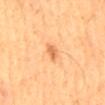{
  "biopsy_status": "not biopsied; imaged during a skin examination",
  "patient": {
    "sex": "male",
    "age_approx": 60
  },
  "lighting": "cross-polarized",
  "site": "back",
  "automated_metrics": {
    "area_mm2_approx": 2.5,
    "cielab_L": 66,
    "cielab_a": 27,
    "cielab_b": 44,
    "vs_skin_contrast_norm": 7.0,
    "border_irregularity_0_10": 2.0,
    "color_variation_0_10": 1.5,
    "peripheral_color_asymmetry": 0.5,
    "lesion_detection_confidence_0_100": 100
  },
  "image": {
    "source": "total-body photography crop",
    "field_of_view_mm": 15
  },
  "lesion_size": {
    "long_diameter_mm_approx": 2.5
  }
}